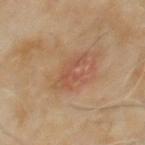Clinical impression: This lesion was catalogued during total-body skin photography and was not selected for biopsy. Image and clinical context: Imaged with cross-polarized lighting. Automated tile analysis of the lesion measured an area of roughly 5 mm², an outline eccentricity of about 0.85 (0 = round, 1 = elongated), and two-axis asymmetry of about 0.5. The software also gave a lesion color around L≈49 a*≈22 b*≈30 in CIELAB and a normalized border contrast of about 5. It also reported a nevus-likeness score of about 5/100. Approximately 4 mm at its widest. The lesion is on the mid back. A region of skin cropped from a whole-body photographic capture, roughly 15 mm wide. The patient is a male aged 58 to 62.